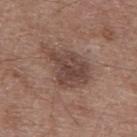Impression:
Imaged during a routine full-body skin examination; the lesion was not biopsied and no histopathology is available.
Background:
A roughly 15 mm field-of-view crop from a total-body skin photograph. About 6.5 mm across. The tile uses white-light illumination. A male patient, aged approximately 60. From the upper back. Automated image analysis of the tile measured border irregularity of about 4.5 on a 0–10 scale and radial color variation of about 1.5.